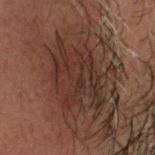Notes:
- notes: catalogued during a skin exam; not biopsied
- acquisition: ~15 mm crop, total-body skin-cancer survey
- anatomic site: the head or neck
- patient: male, in their 70s